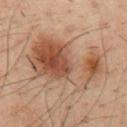| feature | finding |
|---|---|
| workup | no biopsy performed (imaged during a skin exam) |
| body site | the chest |
| size | ≈9 mm |
| lighting | cross-polarized illumination |
| automated lesion analysis | a lesion area of about 36 mm², an eccentricity of roughly 0.8, and a shape-asymmetry score of about 0.35 (0 = symmetric); a lesion color around L≈47 a*≈19 b*≈29 in CIELAB and a normalized lesion–skin contrast near 8; a within-lesion color-variation index near 10/10; a detector confidence of about 100 out of 100 that the crop contains a lesion |
| image source | total-body-photography crop, ~15 mm field of view |
| patient | male, approximately 35 years of age |